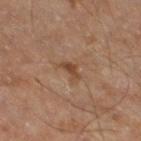Q: Is there a histopathology result?
A: imaged on a skin check; not biopsied
Q: What kind of image is this?
A: 15 mm crop, total-body photography
Q: What is the anatomic site?
A: the right thigh
Q: Who is the patient?
A: male, roughly 70 years of age